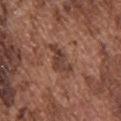Case summary:
- biopsy status · imaged on a skin check; not biopsied
- imaging modality · total-body-photography crop, ~15 mm field of view
- lesion size · about 4.5 mm
- illumination · white-light
- body site · the upper back
- patient · male, aged approximately 75